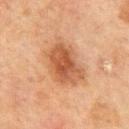Assessment:
Part of a total-body skin-imaging series; this lesion was reviewed on a skin check and was not flagged for biopsy.
Background:
Captured under cross-polarized illumination. The lesion is on the mid back. About 6 mm across. A 15 mm close-up tile from a total-body photography series done for melanoma screening. An algorithmic analysis of the crop reported a lesion area of about 18 mm² and an outline eccentricity of about 0.8 (0 = round, 1 = elongated). The analysis additionally found a lesion color around L≈46 a*≈22 b*≈32 in CIELAB, about 10 CIELAB-L* units darker than the surrounding skin, and a normalized border contrast of about 8. And it measured a border-irregularity index near 2.5/10 and a within-lesion color-variation index near 5/10. The software also gave a detector confidence of about 100 out of 100 that the crop contains a lesion. The subject is a male aged around 70.